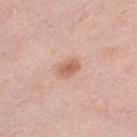Q: Is there a histopathology result?
A: no biopsy performed (imaged during a skin exam)
Q: What are the patient's age and sex?
A: female, in their 40s
Q: How large is the lesion?
A: ≈2.5 mm
Q: What kind of image is this?
A: ~15 mm tile from a whole-body skin photo
Q: Lesion location?
A: the left thigh